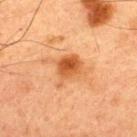Imaged during a routine full-body skin examination; the lesion was not biopsied and no histopathology is available. Imaged with cross-polarized lighting. A male subject aged approximately 60. A 15 mm close-up extracted from a 3D total-body photography capture. From the upper back.